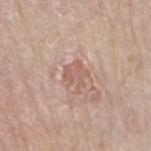Captured during whole-body skin photography for melanoma surveillance; the lesion was not biopsied. The lesion is on the right forearm. A lesion tile, about 15 mm wide, cut from a 3D total-body photograph. Captured under white-light illumination. The subject is a male aged approximately 65.